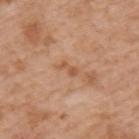The lesion was tiled from a total-body skin photograph and was not biopsied. A male subject aged 63–67. From the upper back. A roughly 15 mm field-of-view crop from a total-body skin photograph.